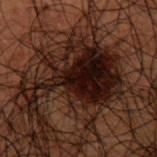Recorded during total-body skin imaging; not selected for excision or biopsy. A male patient roughly 50 years of age. A close-up tile cropped from a whole-body skin photograph, about 15 mm across. This is a cross-polarized tile. An algorithmic analysis of the crop reported a lesion color around L≈11 a*≈13 b*≈13 in CIELAB and roughly 10 lightness units darker than nearby skin. About 10.5 mm across. From the upper back.